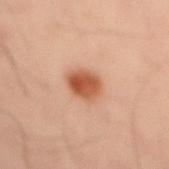This lesion was catalogued during total-body skin photography and was not selected for biopsy.
The lesion is located on the back.
A male subject, about 50 years old.
A 15 mm crop from a total-body photograph taken for skin-cancer surveillance.